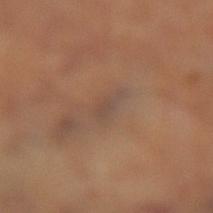workup: imaged on a skin check; not biopsied
illumination: cross-polarized illumination
image-analysis metrics: a lesion area of about 3 mm² and a shape eccentricity near 0.9; a mean CIELAB color near L≈48 a*≈17 b*≈26 and a normalized border contrast of about 5; a color-variation rating of about 1.5/10 and radial color variation of about 0.5
location: the left lower leg
acquisition: ~15 mm crop, total-body skin-cancer survey
diameter: about 3 mm